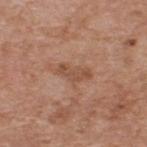Context:
The lesion is located on the upper back. This image is a 15 mm lesion crop taken from a total-body photograph. Imaged with white-light lighting. A male subject, about 60 years old.